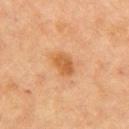No biopsy was performed on this lesion — it was imaged during a full skin examination and was not determined to be concerning. This image is a 15 mm lesion crop taken from a total-body photograph. On the right upper arm. Longest diameter approximately 3 mm. The tile uses cross-polarized illumination. The patient is a female approximately 70 years of age.